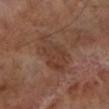The lesion was tiled from a total-body skin photograph and was not biopsied. The lesion-visualizer software estimated an area of roughly 16 mm², a shape eccentricity near 0.8, and a symmetry-axis asymmetry near 0.25. The software also gave a mean CIELAB color near L≈37 a*≈19 b*≈25, roughly 6 lightness units darker than nearby skin, and a normalized lesion–skin contrast near 6. The analysis additionally found a border-irregularity index near 3.5/10, internal color variation of about 3.5 on a 0–10 scale, and radial color variation of about 1. From the left lower leg. A 15 mm crop from a total-body photograph taken for skin-cancer surveillance. Longest diameter approximately 6.5 mm. A male patient roughly 70 years of age. Imaged with cross-polarized lighting.